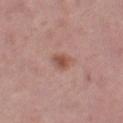Q: Was a biopsy performed?
A: imaged on a skin check; not biopsied
Q: What is the imaging modality?
A: 15 mm crop, total-body photography
Q: Patient demographics?
A: female, aged around 50
Q: Lesion size?
A: about 3 mm
Q: What is the anatomic site?
A: the left thigh